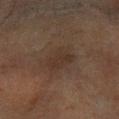- patient · female, aged approximately 60
- image · ~15 mm tile from a whole-body skin photo
- location · the left forearm
- lesion size · about 3.5 mm